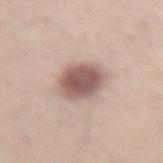Recorded during total-body skin imaging; not selected for excision or biopsy. A region of skin cropped from a whole-body photographic capture, roughly 15 mm wide. The recorded lesion diameter is about 4 mm. Automated tile analysis of the lesion measured a shape eccentricity near 0.35 and a shape-asymmetry score of about 0.1 (0 = symmetric). The analysis additionally found a lesion–skin lightness drop of about 16 and a normalized lesion–skin contrast near 10. On the lower back. A male subject aged 58 to 62.